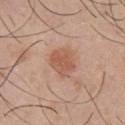Acquisition and patient details: A region of skin cropped from a whole-body photographic capture, roughly 15 mm wide. Captured under white-light illumination. The patient is a male approximately 30 years of age. On the chest.